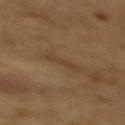workup: catalogued during a skin exam; not biopsied
lesion size: ≈3 mm
body site: the mid back
imaging modality: ~15 mm tile from a whole-body skin photo
subject: male, in their mid- to late 80s or older
illumination: cross-polarized
image-analysis metrics: an outline eccentricity of about 0.95 (0 = round, 1 = elongated) and a symmetry-axis asymmetry near 0.4; a border-irregularity rating of about 5/10, a within-lesion color-variation index near 0/10, and a peripheral color-asymmetry measure near 0; an automated nevus-likeness rating near 0 out of 100